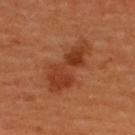The lesion was photographed on a routine skin check and not biopsied; there is no pathology result.
The lesion's longest dimension is about 7 mm.
This is a cross-polarized tile.
A female patient, about 50 years old.
Automated image analysis of the tile measured a lesion color around L≈39 a*≈29 b*≈36 in CIELAB, a lesion–skin lightness drop of about 9, and a normalized border contrast of about 7.5. The analysis additionally found peripheral color asymmetry of about 1.5. It also reported a lesion-detection confidence of about 100/100.
A region of skin cropped from a whole-body photographic capture, roughly 15 mm wide.